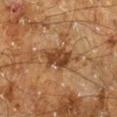notes = imaged on a skin check; not biopsied | image source = total-body-photography crop, ~15 mm field of view | lesion diameter = ~4 mm (longest diameter) | illumination = cross-polarized | body site = the right lower leg | patient = male, about 60 years old.